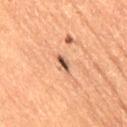Impression:
No biopsy was performed on this lesion — it was imaged during a full skin examination and was not determined to be concerning.
Clinical summary:
The total-body-photography lesion software estimated an area of roughly 3 mm². It also reported a classifier nevus-likeness of about 0/100 and lesion-presence confidence of about 100/100. Cropped from a whole-body photographic skin survey; the tile spans about 15 mm. Longest diameter approximately 2.5 mm. This is a cross-polarized tile. Located on the right thigh. A female patient approximately 60 years of age.